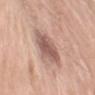workup — imaged on a skin check; not biopsied
imaging modality — 15 mm crop, total-body photography
lesion size — ~4 mm (longest diameter)
patient — female, in their mid- to late 70s
location — the left upper arm
image-analysis metrics — a border-irregularity rating of about 2.5/10, a within-lesion color-variation index near 3.5/10, and a peripheral color-asymmetry measure near 1; a nevus-likeness score of about 20/100